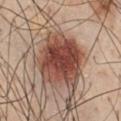Notes:
* subject — male, aged around 60
* anatomic site — the chest
* imaging modality — total-body-photography crop, ~15 mm field of view
* lesion size — ≈6.5 mm
* lighting — white-light